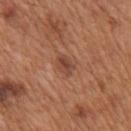Findings:
• notes: total-body-photography surveillance lesion; no biopsy
• patient: male, aged around 65
• acquisition: total-body-photography crop, ~15 mm field of view
• location: the mid back
• illumination: white-light
• size: ~2.5 mm (longest diameter)